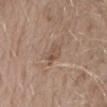This is a white-light tile.
A 15 mm crop from a total-body photograph taken for skin-cancer surveillance.
The lesion is on the right forearm.
The lesion-visualizer software estimated border irregularity of about 2 on a 0–10 scale, a within-lesion color-variation index near 4/10, and a peripheral color-asymmetry measure near 1.5. The analysis additionally found a classifier nevus-likeness of about 0/100 and a lesion-detection confidence of about 100/100.
Approximately 2.5 mm at its widest.
The patient is a male aged around 60.